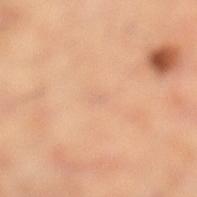Impression: The lesion was photographed on a routine skin check and not biopsied; there is no pathology result. Background: A roughly 15 mm field-of-view crop from a total-body skin photograph. Automated image analysis of the tile measured a lesion area of about 1 mm², a shape eccentricity near 0.85, and a shape-asymmetry score of about 0.4 (0 = symmetric). The software also gave a lesion color around L≈69 a*≈21 b*≈33 in CIELAB, roughly 4 lightness units darker than nearby skin, and a lesion-to-skin contrast of about 3 (normalized; higher = more distinct). The recorded lesion diameter is about 1.5 mm. The patient is a female aged around 60. Located on the right leg. The tile uses cross-polarized illumination.